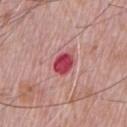Part of a total-body skin-imaging series; this lesion was reviewed on a skin check and was not flagged for biopsy.
About 3 mm across.
From the chest.
A region of skin cropped from a whole-body photographic capture, roughly 15 mm wide.
Captured under white-light illumination.
A male subject approximately 70 years of age.